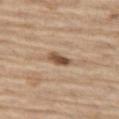Impression:
This lesion was catalogued during total-body skin photography and was not selected for biopsy.
Context:
Automated tile analysis of the lesion measured a normalized border contrast of about 10. And it measured a border-irregularity index near 2.5/10, internal color variation of about 4.5 on a 0–10 scale, and radial color variation of about 1.5. The analysis additionally found a nevus-likeness score of about 90/100 and a detector confidence of about 100 out of 100 that the crop contains a lesion. The lesion's longest dimension is about 3 mm. On the left thigh. The tile uses white-light illumination. A male subject, about 70 years old. This image is a 15 mm lesion crop taken from a total-body photograph.